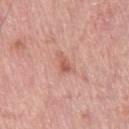The lesion was photographed on a routine skin check and not biopsied; there is no pathology result.
The subject is a male roughly 80 years of age.
A region of skin cropped from a whole-body photographic capture, roughly 15 mm wide.
This is a white-light tile.
Automated tile analysis of the lesion measured a border-irregularity index near 3.5/10 and a peripheral color-asymmetry measure near 0.5. It also reported a detector confidence of about 100 out of 100 that the crop contains a lesion.
Longest diameter approximately 2.5 mm.
The lesion is located on the leg.